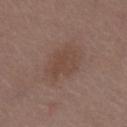Part of a total-body skin-imaging series; this lesion was reviewed on a skin check and was not flagged for biopsy. An algorithmic analysis of the crop reported an area of roughly 11 mm² and an outline eccentricity of about 0.7 (0 = round, 1 = elongated). And it measured a mean CIELAB color near L≈44 a*≈17 b*≈24 and roughly 6 lightness units darker than nearby skin. And it measured a classifier nevus-likeness of about 5/100 and a detector confidence of about 100 out of 100 that the crop contains a lesion. A female patient, aged approximately 50. A 15 mm close-up tile from a total-body photography series done for melanoma screening. From the chest. Captured under white-light illumination. The recorded lesion diameter is about 4.5 mm.